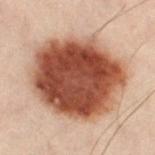Recorded during total-body skin imaging; not selected for excision or biopsy. Located on the left leg. A male subject, roughly 50 years of age. Captured under cross-polarized illumination. Cropped from a total-body skin-imaging series; the visible field is about 15 mm. An algorithmic analysis of the crop reported a lesion color around L≈40 a*≈21 b*≈26 in CIELAB, about 19 CIELAB-L* units darker than the surrounding skin, and a normalized lesion–skin contrast near 14. The analysis additionally found internal color variation of about 6.5 on a 0–10 scale and a peripheral color-asymmetry measure near 2.